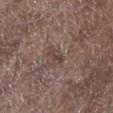- follow-up · imaged on a skin check; not biopsied
- lesion diameter · ~2.5 mm (longest diameter)
- image source · total-body-photography crop, ~15 mm field of view
- subject · male, approximately 70 years of age
- location · the left lower leg
- automated metrics · an average lesion color of about L≈41 a*≈16 b*≈20 (CIELAB), roughly 7 lightness units darker than nearby skin, and a normalized lesion–skin contrast near 6.5; internal color variation of about 0 on a 0–10 scale and a peripheral color-asymmetry measure near 0